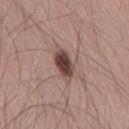subject: male, about 35 years old | anatomic site: the lower back | diameter: about 4 mm | TBP lesion metrics: an area of roughly 7 mm², an eccentricity of roughly 0.85, and a symmetry-axis asymmetry near 0.2; a lesion color around L≈44 a*≈18 b*≈22 in CIELAB, a lesion–skin lightness drop of about 16, and a normalized border contrast of about 11.5; peripheral color asymmetry of about 1; a classifier nevus-likeness of about 100/100 and lesion-presence confidence of about 100/100 | image source: ~15 mm tile from a whole-body skin photo.